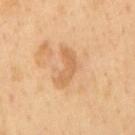Impression: The lesion was tiled from a total-body skin photograph and was not biopsied. Acquisition and patient details: Approximately 4.5 mm at its widest. The patient is a male aged 48–52. A 15 mm close-up extracted from a 3D total-body photography capture. Located on the mid back. Imaged with cross-polarized lighting.